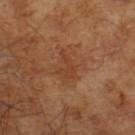Imaged with cross-polarized lighting.
The lesion-visualizer software estimated an average lesion color of about L≈39 a*≈23 b*≈32 (CIELAB), about 6 CIELAB-L* units darker than the surrounding skin, and a lesion-to-skin contrast of about 5 (normalized; higher = more distinct). The software also gave a classifier nevus-likeness of about 10/100.
A 15 mm crop from a total-body photograph taken for skin-cancer surveillance.
The patient is a male roughly 65 years of age.
Measured at roughly 3.5 mm in maximum diameter.